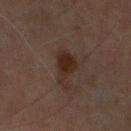A male subject roughly 70 years of age.
Longest diameter approximately 4 mm.
Cropped from a whole-body photographic skin survey; the tile spans about 15 mm.
From the leg.